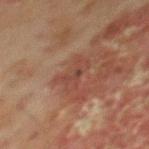Findings:
- workup — no biopsy performed (imaged during a skin exam)
- acquisition — ~15 mm crop, total-body skin-cancer survey
- TBP lesion metrics — an area of roughly 6 mm², an outline eccentricity of about 0.85 (0 = round, 1 = elongated), and two-axis asymmetry of about 0.45; a border-irregularity rating of about 5.5/10, a within-lesion color-variation index near 2.5/10, and radial color variation of about 0.5; a nevus-likeness score of about 0/100 and a detector confidence of about 100 out of 100 that the crop contains a lesion
- patient — male, roughly 70 years of age
- anatomic site — the mid back